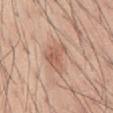biopsy status — no biopsy performed (imaged during a skin exam); tile lighting — white-light; lesion size — ~4 mm (longest diameter); image — ~15 mm crop, total-body skin-cancer survey; body site — the abdomen; subject — male, about 45 years old.